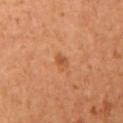Automated image analysis of the tile measured a shape eccentricity near 0.6 and two-axis asymmetry of about 0.3. It also reported a border-irregularity index near 2.5/10. It also reported a detector confidence of about 100 out of 100 that the crop contains a lesion.
A 15 mm crop from a total-body photograph taken for skin-cancer surveillance.
The lesion is located on the arm.
The recorded lesion diameter is about 1.5 mm.
A female patient aged approximately 55.